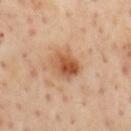No biopsy was performed on this lesion — it was imaged during a full skin examination and was not determined to be concerning.
A male subject, aged 53 to 57.
This image is a 15 mm lesion crop taken from a total-body photograph.
Approximately 3.5 mm at its widest.
From the mid back.